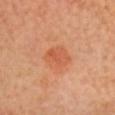Impression: The lesion was tiled from a total-body skin photograph and was not biopsied. Acquisition and patient details: The patient is a female aged around 55. A region of skin cropped from a whole-body photographic capture, roughly 15 mm wide. From the front of the torso. The lesion-visualizer software estimated a lesion area of about 8.5 mm², an eccentricity of roughly 0.55, and a symmetry-axis asymmetry near 0.15. The software also gave an average lesion color of about L≈59 a*≈30 b*≈39 (CIELAB) and roughly 7 lightness units darker than nearby skin. And it measured an automated nevus-likeness rating near 35 out of 100. The recorded lesion diameter is about 3.5 mm.